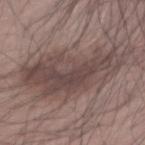Impression:
Imaged during a routine full-body skin examination; the lesion was not biopsied and no histopathology is available.
Clinical summary:
Automated image analysis of the tile measured two-axis asymmetry of about 0.35. And it measured a lesion color around L≈45 a*≈15 b*≈18 in CIELAB and a normalized lesion–skin contrast near 8. It also reported border irregularity of about 7.5 on a 0–10 scale, a within-lesion color-variation index near 5/10, and radial color variation of about 2. It also reported a lesion-detection confidence of about 65/100. A region of skin cropped from a whole-body photographic capture, roughly 15 mm wide. The lesion's longest dimension is about 12 mm. From the left arm. A male patient aged 68–72.